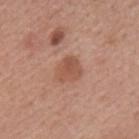| key | value |
|---|---|
| patient | male, aged 63–67 |
| lesion size | about 3 mm |
| site | the mid back |
| tile lighting | white-light illumination |
| acquisition | 15 mm crop, total-body photography |
| TBP lesion metrics | a lesion area of about 6.5 mm² and an outline eccentricity of about 0.5 (0 = round, 1 = elongated) |